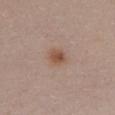Impression: Captured during whole-body skin photography for melanoma surveillance; the lesion was not biopsied. Acquisition and patient details: A 15 mm close-up extracted from a 3D total-body photography capture. The lesion is located on the chest. Longest diameter approximately 2.5 mm. Imaged with white-light lighting. A female patient about 30 years old.